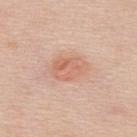lesion size = ≈4.5 mm | imaging modality = total-body-photography crop, ~15 mm field of view | anatomic site = the upper back | lighting = white-light illumination | patient = female, aged approximately 45 | image-analysis metrics = a mean CIELAB color near L≈64 a*≈23 b*≈31, roughly 8 lightness units darker than nearby skin, and a normalized lesion–skin contrast near 6.